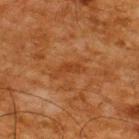Q: Is there a histopathology result?
A: no biopsy performed (imaged during a skin exam)
Q: What are the patient's age and sex?
A: male, roughly 65 years of age
Q: Lesion size?
A: ≈3.5 mm
Q: How was this image acquired?
A: 15 mm crop, total-body photography
Q: Lesion location?
A: the upper back
Q: What lighting was used for the tile?
A: cross-polarized